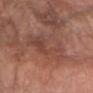Assessment: Imaged during a routine full-body skin examination; the lesion was not biopsied and no histopathology is available. Acquisition and patient details: The subject is a female aged around 80. Imaged with white-light lighting. Measured at roughly 7 mm in maximum diameter. Automated image analysis of the tile measured an outline eccentricity of about 0.85 (0 = round, 1 = elongated) and two-axis asymmetry of about 0.3. It also reported border irregularity of about 4 on a 0–10 scale, a within-lesion color-variation index near 5.5/10, and a peripheral color-asymmetry measure near 2. The analysis additionally found a classifier nevus-likeness of about 0/100 and a detector confidence of about 75 out of 100 that the crop contains a lesion. Located on the left forearm. A 15 mm close-up extracted from a 3D total-body photography capture.